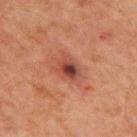patient:
  sex: male
  age_approx: 65
image:
  source: total-body photography crop
  field_of_view_mm: 15
site: chest